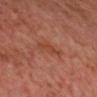subject: male, about 60 years old
lighting: cross-polarized illumination
anatomic site: the head or neck
diameter: about 3.5 mm
image source: total-body-photography crop, ~15 mm field of view